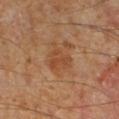Clinical impression:
This lesion was catalogued during total-body skin photography and was not selected for biopsy.
Context:
Automated image analysis of the tile measured a border-irregularity rating of about 3/10, internal color variation of about 3 on a 0–10 scale, and a peripheral color-asymmetry measure near 1. The analysis additionally found an automated nevus-likeness rating near 0 out of 100 and a lesion-detection confidence of about 100/100. A 15 mm close-up extracted from a 3D total-body photography capture. A male subject approximately 60 years of age. The recorded lesion diameter is about 3 mm. The lesion is located on the right lower leg. Captured under cross-polarized illumination.